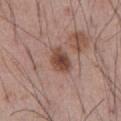Assessment: The lesion was photographed on a routine skin check and not biopsied; there is no pathology result. Acquisition and patient details: The subject is a male about 55 years old. Located on the abdomen. The lesion's longest dimension is about 3.5 mm. A lesion tile, about 15 mm wide, cut from a 3D total-body photograph. Automated image analysis of the tile measured a lesion area of about 6.5 mm², a shape eccentricity near 0.7, and a shape-asymmetry score of about 0.2 (0 = symmetric). It also reported a lesion color around L≈46 a*≈21 b*≈25 in CIELAB, a lesion–skin lightness drop of about 13, and a lesion-to-skin contrast of about 9.5 (normalized; higher = more distinct). And it measured a border-irregularity rating of about 2/10, a color-variation rating of about 4/10, and a peripheral color-asymmetry measure near 1. Captured under white-light illumination.